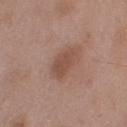No biopsy was performed on this lesion — it was imaged during a full skin examination and was not determined to be concerning. The tile uses white-light illumination. From the left upper arm. The lesion-visualizer software estimated an average lesion color of about L≈49 a*≈20 b*≈28 (CIELAB), about 8 CIELAB-L* units darker than the surrounding skin, and a normalized lesion–skin contrast near 6.5. The analysis additionally found an automated nevus-likeness rating near 40 out of 100. A male patient, in their 50s. A lesion tile, about 15 mm wide, cut from a 3D total-body photograph.